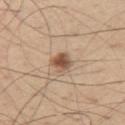The lesion was photographed on a routine skin check and not biopsied; there is no pathology result. A 15 mm close-up extracted from a 3D total-body photography capture. The lesion is located on the left upper arm. The subject is a male about 55 years old. This is a white-light tile. Measured at roughly 3 mm in maximum diameter.